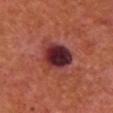The lesion was photographed on a routine skin check and not biopsied; there is no pathology result. Cropped from a total-body skin-imaging series; the visible field is about 15 mm. Captured under cross-polarized illumination. Measured at roughly 4.5 mm in maximum diameter. An algorithmic analysis of the crop reported an area of roughly 14 mm² and a symmetry-axis asymmetry near 0.2. The analysis additionally found a lesion color around L≈33 a*≈29 b*≈21 in CIELAB and a lesion-to-skin contrast of about 15 (normalized; higher = more distinct). It also reported a border-irregularity index near 2.5/10, a color-variation rating of about 10/10, and a peripheral color-asymmetry measure near 4. The analysis additionally found a classifier nevus-likeness of about 50/100 and lesion-presence confidence of about 100/100. A female subject about 55 years old. Located on the chest.